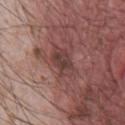Part of a total-body skin-imaging series; this lesion was reviewed on a skin check and was not flagged for biopsy.
A male patient approximately 65 years of age.
Approximately 4 mm at its widest.
The total-body-photography lesion software estimated a lesion-to-skin contrast of about 7 (normalized; higher = more distinct). The software also gave border irregularity of about 2.5 on a 0–10 scale and peripheral color asymmetry of about 1.5. And it measured an automated nevus-likeness rating near 0 out of 100.
Imaged with white-light lighting.
Located on the front of the torso.
A lesion tile, about 15 mm wide, cut from a 3D total-body photograph.